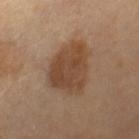Clinical impression: The lesion was photographed on a routine skin check and not biopsied; there is no pathology result. Image and clinical context: The lesion is on the right thigh. A 15 mm close-up extracted from a 3D total-body photography capture. This is a cross-polarized tile. A female subject approximately 65 years of age.